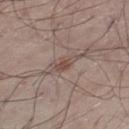  biopsy_status: not biopsied; imaged during a skin examination
  patient:
    sex: male
    age_approx: 55
  site: left thigh
  automated_metrics:
    area_mm2_approx: 3.5
    eccentricity: 0.85
    shape_asymmetry: 0.3
    border_irregularity_0_10: 3.5
    color_variation_0_10: 1.0
    peripheral_color_asymmetry: 0.0
  image:
    source: total-body photography crop
    field_of_view_mm: 15
  lesion_size:
    long_diameter_mm_approx: 3.0
  lighting: white-light